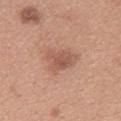Impression: Recorded during total-body skin imaging; not selected for excision or biopsy. Context: A lesion tile, about 15 mm wide, cut from a 3D total-body photograph. This is a white-light tile. An algorithmic analysis of the crop reported an area of roughly 8.5 mm², an eccentricity of roughly 0.65, and a shape-asymmetry score of about 0.3 (0 = symmetric). And it measured a border-irregularity index near 3.5/10, a color-variation rating of about 3/10, and a peripheral color-asymmetry measure near 1. The lesion is located on the upper back. The subject is a female aged 28–32. Approximately 3.5 mm at its widest.